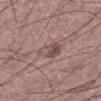No biopsy was performed on this lesion — it was imaged during a full skin examination and was not determined to be concerning.
A close-up tile cropped from a whole-body skin photograph, about 15 mm across.
A male subject about 60 years old.
The lesion is located on the abdomen.